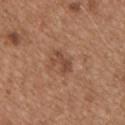A male subject aged approximately 65. The lesion's longest dimension is about 3 mm. Imaged with white-light lighting. On the chest. A close-up tile cropped from a whole-body skin photograph, about 15 mm across.